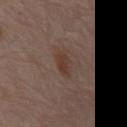Q: Was a biopsy performed?
A: imaged on a skin check; not biopsied
Q: Who is the patient?
A: male, aged 78 to 82
Q: What is the lesion's diameter?
A: ~3 mm (longest diameter)
Q: Lesion location?
A: the abdomen
Q: What kind of image is this?
A: ~15 mm crop, total-body skin-cancer survey